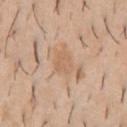imaging modality = total-body-photography crop, ~15 mm field of view | diameter = about 2.5 mm | automated lesion analysis = a lesion–skin lightness drop of about 6 and a normalized lesion–skin contrast near 4.5; a border-irregularity index near 2.5/10; a nevus-likeness score of about 0/100 and lesion-presence confidence of about 100/100 | location = the chest | patient = male, about 40 years old.